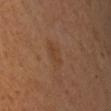{
  "biopsy_status": "not biopsied; imaged during a skin examination",
  "lesion_size": {
    "long_diameter_mm_approx": 2.5
  },
  "image": {
    "source": "total-body photography crop",
    "field_of_view_mm": 15
  },
  "site": "right upper arm",
  "automated_metrics": {
    "border_irregularity_0_10": 4.5,
    "color_variation_0_10": 0.0,
    "peripheral_color_asymmetry": 0.0
  },
  "patient": {
    "sex": "male",
    "age_approx": 50
  }
}